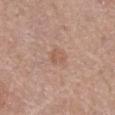biopsy_status: not biopsied; imaged during a skin examination
lighting: white-light
automated_metrics:
  area_mm2_approx: 3.5
  eccentricity: 0.7
  shape_asymmetry: 0.35
site: left lower leg
lesion_size:
  long_diameter_mm_approx: 2.5
patient:
  sex: female
  age_approx: 60
image:
  source: total-body photography crop
  field_of_view_mm: 15